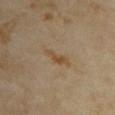Assessment:
Captured during whole-body skin photography for melanoma surveillance; the lesion was not biopsied.
Clinical summary:
The lesion's longest dimension is about 4 mm. The lesion is located on the chest. The subject is a female aged approximately 35. Automated tile analysis of the lesion measured a lesion area of about 4 mm², a shape eccentricity near 0.95, and a symmetry-axis asymmetry near 0.4. The analysis additionally found a lesion color around L≈46 a*≈14 b*≈32 in CIELAB. The software also gave a within-lesion color-variation index near 0.5/10 and radial color variation of about 0. It also reported an automated nevus-likeness rating near 10 out of 100. Imaged with cross-polarized lighting. A region of skin cropped from a whole-body photographic capture, roughly 15 mm wide.